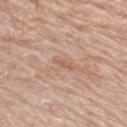  biopsy_status: not biopsied; imaged during a skin examination
  image:
    source: total-body photography crop
    field_of_view_mm: 15
  site: upper back
  patient:
    sex: male
    age_approx: 80